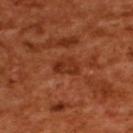This lesion was catalogued during total-body skin photography and was not selected for biopsy.
The lesion is located on the upper back.
The patient is a female aged around 55.
The total-body-photography lesion software estimated an automated nevus-likeness rating near 0 out of 100.
The tile uses cross-polarized illumination.
A 15 mm close-up extracted from a 3D total-body photography capture.